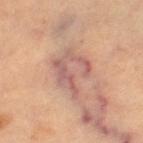{
  "biopsy_status": "not biopsied; imaged during a skin examination",
  "lighting": "cross-polarized",
  "site": "left thigh",
  "patient": {
    "age_approx": 60
  },
  "image": {
    "source": "total-body photography crop",
    "field_of_view_mm": 15
  },
  "lesion_size": {
    "long_diameter_mm_approx": 5.0
  },
  "automated_metrics": {
    "eccentricity": 0.65,
    "shape_asymmetry": 0.5,
    "peripheral_color_asymmetry": 1.0,
    "nevus_likeness_0_100": 0,
    "lesion_detection_confidence_0_100": 70
  }
}